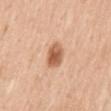| key | value |
|---|---|
| image | ~15 mm tile from a whole-body skin photo |
| illumination | white-light |
| body site | the back |
| size | ≈3 mm |
| subject | male, aged approximately 50 |